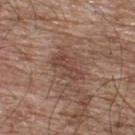The lesion was photographed on a routine skin check and not biopsied; there is no pathology result. From the back. A male patient, roughly 65 years of age. A close-up tile cropped from a whole-body skin photograph, about 15 mm across. Automated tile analysis of the lesion measured an average lesion color of about L≈44 a*≈20 b*≈25 (CIELAB) and a lesion-to-skin contrast of about 6 (normalized; higher = more distinct). It also reported a border-irregularity rating of about 4.5/10. It also reported a classifier nevus-likeness of about 0/100. This is a white-light tile.